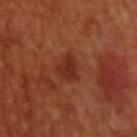Q: Illumination type?
A: cross-polarized illumination
Q: What is the lesion's diameter?
A: ≈3 mm
Q: Lesion location?
A: the upper back
Q: Patient demographics?
A: male, roughly 50 years of age
Q: What is the imaging modality?
A: ~15 mm tile from a whole-body skin photo